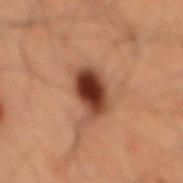Clinical impression: No biopsy was performed on this lesion — it was imaged during a full skin examination and was not determined to be concerning. Image and clinical context: The lesion is located on the back. Cropped from a whole-body photographic skin survey; the tile spans about 15 mm. Imaged with cross-polarized lighting. A male patient, aged 58–62.